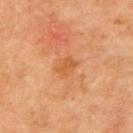Captured during whole-body skin photography for melanoma surveillance; the lesion was not biopsied.
A 15 mm crop from a total-body photograph taken for skin-cancer surveillance.
On the arm.
The tile uses cross-polarized illumination.
The lesion's longest dimension is about 3 mm.
A female patient aged approximately 70.